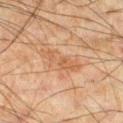Case summary:
– notes — total-body-photography surveillance lesion; no biopsy
– image — total-body-photography crop, ~15 mm field of view
– site — the right lower leg
– patient — male, aged approximately 45
– size — about 5 mm